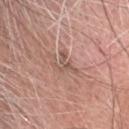biopsy status: catalogued during a skin exam; not biopsied
size: ≈4 mm
subject: male, about 60 years old
location: the head or neck
image: ~15 mm crop, total-body skin-cancer survey
automated lesion analysis: a border-irregularity rating of about 9/10, a color-variation rating of about 0/10, and radial color variation of about 0